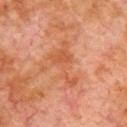This lesion was catalogued during total-body skin photography and was not selected for biopsy.
The lesion is located on the chest.
A male patient, aged 78 to 82.
A 15 mm close-up tile from a total-body photography series done for melanoma screening.